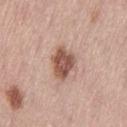<tbp_lesion>
<biopsy_status>not biopsied; imaged during a skin examination</biopsy_status>
<site>left thigh</site>
<image>
  <source>total-body photography crop</source>
  <field_of_view_mm>15</field_of_view_mm>
</image>
<patient>
  <sex>female</sex>
  <age_approx>65</age_approx>
</patient>
</tbp_lesion>